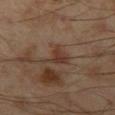<lesion>
  <biopsy_status>not biopsied; imaged during a skin examination</biopsy_status>
  <lesion_size>
    <long_diameter_mm_approx>3.5</long_diameter_mm_approx>
  </lesion_size>
  <patient>
    <sex>male</sex>
    <age_approx>55</age_approx>
  </patient>
  <lighting>cross-polarized</lighting>
  <site>left thigh</site>
  <image>
    <source>total-body photography crop</source>
    <field_of_view_mm>15</field_of_view_mm>
  </image>
</lesion>